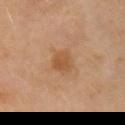biopsy status = no biopsy performed (imaged during a skin exam)
image-analysis metrics = two-axis asymmetry of about 0.2; a mean CIELAB color near L≈51 a*≈21 b*≈36, a lesion–skin lightness drop of about 8, and a normalized border contrast of about 6.5
patient = female, in their 50s
body site = the left upper arm
illumination = cross-polarized illumination
diameter = ~3 mm (longest diameter)
image = total-body-photography crop, ~15 mm field of view